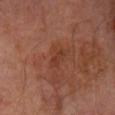The lesion was tiled from a total-body skin photograph and was not biopsied. The patient is a male roughly 70 years of age. A 15 mm close-up extracted from a 3D total-body photography capture. The lesion is located on the right lower leg.